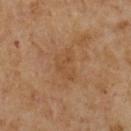Clinical impression: Recorded during total-body skin imaging; not selected for excision or biopsy. Clinical summary: The patient is a male aged approximately 65. Cropped from a whole-body photographic skin survey; the tile spans about 15 mm. Imaged with cross-polarized lighting. Approximately 3.5 mm at its widest.